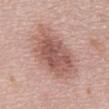{
  "biopsy_status": "not biopsied; imaged during a skin examination",
  "lesion_size": {
    "long_diameter_mm_approx": 7.0
  },
  "patient": {
    "sex": "male",
    "age_approx": 70
  },
  "site": "mid back",
  "image": {
    "source": "total-body photography crop",
    "field_of_view_mm": 15
  },
  "automated_metrics": {
    "nevus_likeness_0_100": 35,
    "lesion_detection_confidence_0_100": 100
  },
  "lighting": "white-light"
}